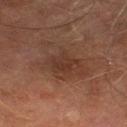Recorded during total-body skin imaging; not selected for excision or biopsy. A roughly 15 mm field-of-view crop from a total-body skin photograph. A male subject, in their mid- to late 70s. The lesion is on the left thigh.